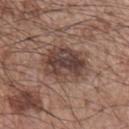This lesion was catalogued during total-body skin photography and was not selected for biopsy. From the left upper arm. The patient is a male about 65 years old. A lesion tile, about 15 mm wide, cut from a 3D total-body photograph. Longest diameter approximately 5 mm. The lesion-visualizer software estimated a border-irregularity rating of about 2.5/10, internal color variation of about 6.5 on a 0–10 scale, and a peripheral color-asymmetry measure near 2.5. The software also gave a lesion-detection confidence of about 100/100.